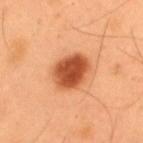  biopsy_status: not biopsied; imaged during a skin examination
  image:
    source: total-body photography crop
    field_of_view_mm: 15
  patient:
    sex: male
    age_approx: 55
  site: upper back